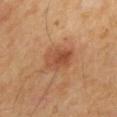Case summary:
* notes — imaged on a skin check; not biopsied
* acquisition — ~15 mm crop, total-body skin-cancer survey
* automated metrics — a border-irregularity rating of about 2/10 and a color-variation rating of about 5/10; a classifier nevus-likeness of about 75/100
* site — the back
* subject — male, aged approximately 55
* lighting — cross-polarized